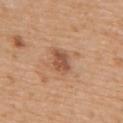Recorded during total-body skin imaging; not selected for excision or biopsy.
On the upper back.
Approximately 3 mm at its widest.
A male subject aged 68 to 72.
A roughly 15 mm field-of-view crop from a total-body skin photograph.